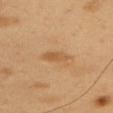Recorded during total-body skin imaging; not selected for excision or biopsy.
The lesion is located on the right upper arm.
This is a cross-polarized tile.
This image is a 15 mm lesion crop taken from a total-body photograph.
The subject is a male approximately 50 years of age.